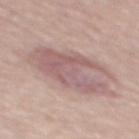notes: total-body-photography surveillance lesion; no biopsy
patient: male, aged around 80
size: about 8 mm
image source: total-body-photography crop, ~15 mm field of view
site: the mid back